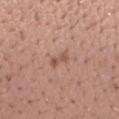Case summary:
- workup · total-body-photography surveillance lesion; no biopsy
- lighting · white-light illumination
- imaging modality · ~15 mm crop, total-body skin-cancer survey
- location · the left forearm
- size · ~2.5 mm (longest diameter)
- patient · male, roughly 40 years of age